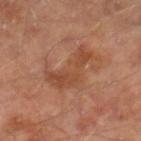Findings:
- notes: no biopsy performed (imaged during a skin exam)
- anatomic site: the right lower leg
- lighting: cross-polarized
- lesion diameter: about 6 mm
- subject: male, about 65 years old
- imaging modality: 15 mm crop, total-body photography